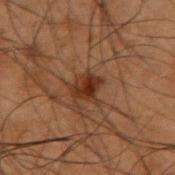{
  "biopsy_status": "not biopsied; imaged during a skin examination",
  "image": {
    "source": "total-body photography crop",
    "field_of_view_mm": 15
  },
  "patient": {
    "sex": "male",
    "age_approx": 50
  },
  "lighting": "cross-polarized",
  "site": "right upper arm",
  "automated_metrics": {
    "border_irregularity_0_10": 3.5,
    "color_variation_0_10": 4.0,
    "peripheral_color_asymmetry": 1.5
  }
}